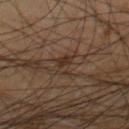Captured during whole-body skin photography for melanoma surveillance; the lesion was not biopsied.
A region of skin cropped from a whole-body photographic capture, roughly 15 mm wide.
Automated image analysis of the tile measured an area of roughly 3.5 mm² and two-axis asymmetry of about 0.45. And it measured a border-irregularity index near 4/10 and a color-variation rating of about 3.5/10. The analysis additionally found a nevus-likeness score of about 0/100 and a detector confidence of about 90 out of 100 that the crop contains a lesion.
Captured under cross-polarized illumination.
A male patient, roughly 70 years of age.
On the right thigh.
Approximately 2.5 mm at its widest.